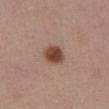<case>
  <biopsy_status>not biopsied; imaged during a skin examination</biopsy_status>
  <automated_metrics>
    <area_mm2_approx>6.0</area_mm2_approx>
    <eccentricity>0.55</eccentricity>
    <shape_asymmetry>0.1</shape_asymmetry>
  </automated_metrics>
  <image>
    <source>total-body photography crop</source>
    <field_of_view_mm>15</field_of_view_mm>
  </image>
  <lighting>white-light</lighting>
  <site>abdomen</site>
  <patient>
    <sex>female</sex>
    <age_approx>55</age_approx>
  </patient>
</case>